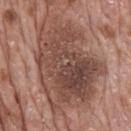notes: imaged on a skin check; not biopsied
illumination: white-light illumination
lesion size: about 11.5 mm
subject: male, about 70 years old
image source: 15 mm crop, total-body photography
body site: the mid back